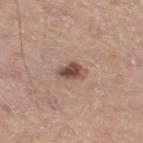  biopsy_status: not biopsied; imaged during a skin examination
  patient:
    sex: male
    age_approx: 65
  lesion_size:
    long_diameter_mm_approx: 3.5
  lighting: white-light
  image:
    source: total-body photography crop
    field_of_view_mm: 15
  site: left lower leg